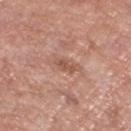Findings:
– workup: total-body-photography surveillance lesion; no biopsy
– patient: female, aged around 70
– image source: 15 mm crop, total-body photography
– body site: the left lower leg
– lesion diameter: ~2.5 mm (longest diameter)
– lighting: white-light illumination
– automated metrics: a symmetry-axis asymmetry near 0.3; a mean CIELAB color near L≈53 a*≈23 b*≈29, a lesion–skin lightness drop of about 9, and a lesion-to-skin contrast of about 6.5 (normalized; higher = more distinct); border irregularity of about 3.5 on a 0–10 scale and radial color variation of about 0